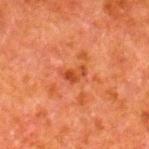The lesion is on the leg.
This image is a 15 mm lesion crop taken from a total-body photograph.
The patient is a male approximately 80 years of age.